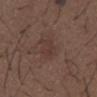  biopsy_status: not biopsied; imaged during a skin examination
  image:
    source: total-body photography crop
    field_of_view_mm: 15
  lesion_size:
    long_diameter_mm_approx: 4.0
  automated_metrics:
    border_irregularity_0_10: 3.5
    color_variation_0_10: 3.0
    peripheral_color_asymmetry: 1.0
    lesion_detection_confidence_0_100: 100
  site: mid back
  lighting: white-light
  patient:
    sex: male
    age_approx: 50